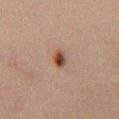Notes:
– workup: catalogued during a skin exam; not biopsied
– tile lighting: cross-polarized
– patient: male, aged 28 to 32
– image: 15 mm crop, total-body photography
– diameter: about 2.5 mm
– anatomic site: the front of the torso